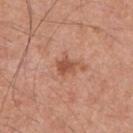Captured during whole-body skin photography for melanoma surveillance; the lesion was not biopsied.
A roughly 15 mm field-of-view crop from a total-body skin photograph.
A male subject aged around 55.
From the chest.
Approximately 2.5 mm at its widest.
Imaged with white-light lighting.